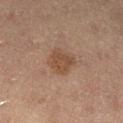follow-up = no biopsy performed (imaged during a skin exam).